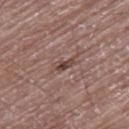  biopsy_status: not biopsied; imaged during a skin examination
  lesion_size:
    long_diameter_mm_approx: 2.5
  lighting: white-light
  site: leg
  image:
    source: total-body photography crop
    field_of_view_mm: 15
  patient:
    sex: male
    age_approx: 80
  automated_metrics:
    color_variation_0_10: 2.0
    nevus_likeness_0_100: 5
    lesion_detection_confidence_0_100: 95